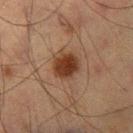Impression:
The lesion was photographed on a routine skin check and not biopsied; there is no pathology result.
Context:
The total-body-photography lesion software estimated a shape-asymmetry score of about 0.1 (0 = symmetric). It also reported a peripheral color-asymmetry measure near 1. On the left thigh. The lesion's longest dimension is about 3.5 mm. Imaged with cross-polarized lighting. A region of skin cropped from a whole-body photographic capture, roughly 15 mm wide. The patient is a male aged 63 to 67.